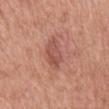Recorded during total-body skin imaging; not selected for excision or biopsy. From the mid back. Cropped from a total-body skin-imaging series; the visible field is about 15 mm. A male subject roughly 70 years of age.